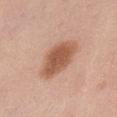Q: Was this lesion biopsied?
A: catalogued during a skin exam; not biopsied
Q: What lighting was used for the tile?
A: white-light
Q: Lesion size?
A: about 5.5 mm
Q: Lesion location?
A: the abdomen
Q: Patient demographics?
A: female, aged 48–52
Q: What did automated image analysis measure?
A: a lesion area of about 14 mm², a shape eccentricity near 0.8, and a symmetry-axis asymmetry near 0.15; an automated nevus-likeness rating near 100 out of 100 and a detector confidence of about 100 out of 100 that the crop contains a lesion
Q: What kind of image is this?
A: 15 mm crop, total-body photography